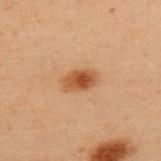Impression: Part of a total-body skin-imaging series; this lesion was reviewed on a skin check and was not flagged for biopsy. Context: The tile uses cross-polarized illumination. About 3.5 mm across. The lesion-visualizer software estimated a lesion area of about 6 mm² and a symmetry-axis asymmetry near 0.15. It also reported a lesion color around L≈45 a*≈22 b*≈34 in CIELAB and roughly 11 lightness units darker than nearby skin. The analysis additionally found a color-variation rating of about 4/10 and radial color variation of about 1.5. A female subject, aged approximately 50. A close-up tile cropped from a whole-body skin photograph, about 15 mm across. The lesion is located on the back.